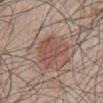<tbp_lesion>
<biopsy_status>not biopsied; imaged during a skin examination</biopsy_status>
<image>
  <source>total-body photography crop</source>
  <field_of_view_mm>15</field_of_view_mm>
</image>
<site>abdomen</site>
<automated_metrics>
  <area_mm2_approx>12.0</area_mm2_approx>
  <eccentricity>0.7</eccentricity>
  <shape_asymmetry>0.4</shape_asymmetry>
  <cielab_L>51</cielab_L>
  <cielab_a>20</cielab_a>
  <cielab_b>24</cielab_b>
  <vs_skin_darker_L>8.0</vs_skin_darker_L>
  <vs_skin_contrast_norm>6.0</vs_skin_contrast_norm>
  <border_irregularity_0_10>4.5</border_irregularity_0_10>
</automated_metrics>
<lesion_size>
  <long_diameter_mm_approx>5.5</long_diameter_mm_approx>
</lesion_size>
<lighting>white-light</lighting>
<patient>
  <sex>male</sex>
  <age_approx>50</age_approx>
</patient>
</tbp_lesion>